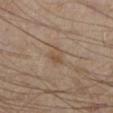<case>
<biopsy_status>not biopsied; imaged during a skin examination</biopsy_status>
<lesion_size>
  <long_diameter_mm_approx>2.5</long_diameter_mm_approx>
</lesion_size>
<automated_metrics>
  <area_mm2_approx>4.0</area_mm2_approx>
  <eccentricity>0.6</eccentricity>
  <nevus_likeness_0_100>0</nevus_likeness_0_100>
  <lesion_detection_confidence_0_100>100</lesion_detection_confidence_0_100>
</automated_metrics>
<patient>
  <sex>male</sex>
  <age_approx>45</age_approx>
</patient>
<lighting>cross-polarized</lighting>
<image>
  <source>total-body photography crop</source>
  <field_of_view_mm>15</field_of_view_mm>
</image>
<site>leg</site>
</case>